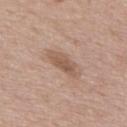follow-up: catalogued during a skin exam; not biopsied | acquisition: ~15 mm tile from a whole-body skin photo | image-analysis metrics: an automated nevus-likeness rating near 0 out of 100 and a detector confidence of about 100 out of 100 that the crop contains a lesion | lesion diameter: ~4 mm (longest diameter) | patient: male, aged approximately 55.